The lesion is located on the front of the torso · longest diameter approximately 9 mm · captured under cross-polarized illumination · a 15 mm close-up extracted from a 3D total-body photography capture · a male patient, in their mid-60s.
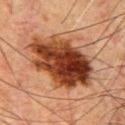histopathologic diagnosis = a dysplastic (Clark) nevus — a benign lesion